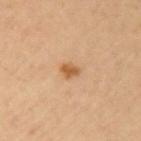subject — male, about 65 years old
anatomic site — the left upper arm
imaging modality — total-body-photography crop, ~15 mm field of view
lesion diameter — ≈2 mm